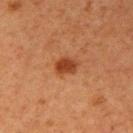Assessment: This lesion was catalogued during total-body skin photography and was not selected for biopsy. Clinical summary: On the right upper arm. A female patient approximately 40 years of age. A roughly 15 mm field-of-view crop from a total-body skin photograph.